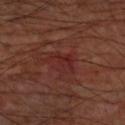Q: Was a biopsy performed?
A: catalogued during a skin exam; not biopsied
Q: Where on the body is the lesion?
A: the left upper arm
Q: Who is the patient?
A: male, roughly 60 years of age
Q: What lighting was used for the tile?
A: cross-polarized illumination
Q: What kind of image is this?
A: total-body-photography crop, ~15 mm field of view
Q: Lesion size?
A: about 4 mm